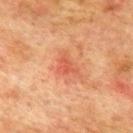Clinical impression: The lesion was tiled from a total-body skin photograph and was not biopsied. Clinical summary: Captured under cross-polarized illumination. The lesion is located on the upper back. A male patient, roughly 75 years of age. Measured at roughly 2.5 mm in maximum diameter. This image is a 15 mm lesion crop taken from a total-body photograph.